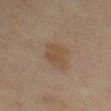follow-up: no biopsy performed (imaged during a skin exam) | tile lighting: cross-polarized | subject: female, aged around 60 | size: about 3.5 mm | site: the left thigh | acquisition: total-body-photography crop, ~15 mm field of view.